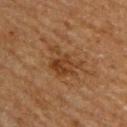No biopsy was performed on this lesion — it was imaged during a full skin examination and was not determined to be concerning. A male patient, approximately 65 years of age. A lesion tile, about 15 mm wide, cut from a 3D total-body photograph. Located on the upper back. This is a cross-polarized tile. The lesion's longest dimension is about 4.5 mm.